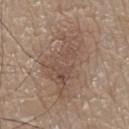Recorded during total-body skin imaging; not selected for excision or biopsy.
Located on the chest.
This is a white-light tile.
A male subject, aged approximately 80.
Longest diameter approximately 9 mm.
A 15 mm close-up extracted from a 3D total-body photography capture.
The lesion-visualizer software estimated a mean CIELAB color near L≈51 a*≈16 b*≈25, roughly 8 lightness units darker than nearby skin, and a normalized border contrast of about 6. The software also gave a nevus-likeness score of about 5/100 and a detector confidence of about 100 out of 100 that the crop contains a lesion.